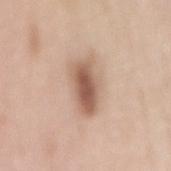biopsy status: total-body-photography surveillance lesion; no biopsy
lighting: white-light
patient: female, aged approximately 70
body site: the mid back
acquisition: 15 mm crop, total-body photography